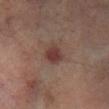Clinical impression:
This lesion was catalogued during total-body skin photography and was not selected for biopsy.
Context:
Captured under cross-polarized illumination. The lesion is located on the right lower leg. About 3 mm across. A male patient, approximately 70 years of age. This image is a 15 mm lesion crop taken from a total-body photograph.